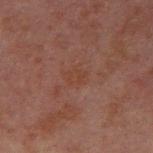workup: total-body-photography surveillance lesion; no biopsy | illumination: cross-polarized | image-analysis metrics: a shape eccentricity near 0.7 and a symmetry-axis asymmetry near 0.3; a lesion color around L≈33 a*≈18 b*≈23 in CIELAB, about 3 CIELAB-L* units darker than the surrounding skin, and a normalized lesion–skin contrast near 5; a border-irregularity index near 3/10, a within-lesion color-variation index near 1.5/10, and peripheral color asymmetry of about 0.5; a nevus-likeness score of about 0/100 and a lesion-detection confidence of about 100/100 | subject: male, in their 30s | diameter: ≈2.5 mm | location: the right upper arm | acquisition: 15 mm crop, total-body photography.